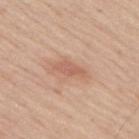| feature | finding |
|---|---|
| follow-up | no biopsy performed (imaged during a skin exam) |
| imaging modality | ~15 mm crop, total-body skin-cancer survey |
| anatomic site | the back |
| patient | male, approximately 45 years of age |
| diameter | about 4 mm |